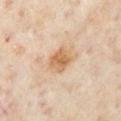Assessment:
The lesion was photographed on a routine skin check and not biopsied; there is no pathology result.
Image and clinical context:
A female subject approximately 60 years of age. Imaged with cross-polarized lighting. The lesion is on the chest. Measured at roughly 3.5 mm in maximum diameter. Cropped from a total-body skin-imaging series; the visible field is about 15 mm.